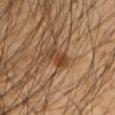follow-up = imaged on a skin check; not biopsied
image source = total-body-photography crop, ~15 mm field of view
automated metrics = a mean CIELAB color near L≈42 a*≈19 b*≈33 and about 9 CIELAB-L* units darker than the surrounding skin; a border-irregularity rating of about 3/10 and a color-variation rating of about 5/10
patient = male, about 45 years old
location = the left forearm
lesion diameter = ≈3.5 mm
lighting = cross-polarized illumination